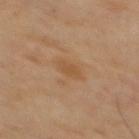Recorded during total-body skin imaging; not selected for excision or biopsy. A 15 mm close-up extracted from a 3D total-body photography capture. The recorded lesion diameter is about 3 mm. The subject is a male aged 68 to 72. Located on the mid back. Imaged with cross-polarized lighting.